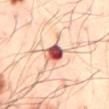follow-up: imaged on a skin check; not biopsied | acquisition: total-body-photography crop, ~15 mm field of view | location: the mid back | patient: male, aged 48 to 52 | tile lighting: cross-polarized illumination.